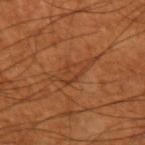workup: catalogued during a skin exam; not biopsied | acquisition: ~15 mm crop, total-body skin-cancer survey | image-analysis metrics: a shape eccentricity near 0.9 and two-axis asymmetry of about 0.5; border irregularity of about 7.5 on a 0–10 scale, internal color variation of about 2.5 on a 0–10 scale, and a peripheral color-asymmetry measure near 1; a nevus-likeness score of about 0/100 | anatomic site: the left upper arm | lighting: cross-polarized illumination | subject: male, in their 60s.